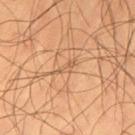Findings:
• image-analysis metrics · a shape eccentricity near 0.9 and a symmetry-axis asymmetry near 0.45; a within-lesion color-variation index near 0/10 and radial color variation of about 0
• subject · male, approximately 60 years of age
• imaging modality · ~15 mm tile from a whole-body skin photo
• diameter · ≈2.5 mm
• tile lighting · cross-polarized illumination
• site · the leg